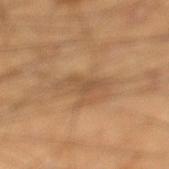Part of a total-body skin-imaging series; this lesion was reviewed on a skin check and was not flagged for biopsy. An algorithmic analysis of the crop reported a footprint of about 5 mm², a shape eccentricity near 0.95, and two-axis asymmetry of about 0.5. It also reported a mean CIELAB color near L≈52 a*≈18 b*≈34, a lesion–skin lightness drop of about 8, and a normalized lesion–skin contrast near 5.5. The software also gave a border-irregularity rating of about 6.5/10, internal color variation of about 1 on a 0–10 scale, and radial color variation of about 0.5. On the left lower leg. Captured under cross-polarized illumination. Longest diameter approximately 4.5 mm. A male patient aged 38–42. A region of skin cropped from a whole-body photographic capture, roughly 15 mm wide.